The lesion is on the chest. A male subject aged 83 or over. The recorded lesion diameter is about 2.5 mm. This image is a 15 mm lesion crop taken from a total-body photograph. The tile uses white-light illumination.
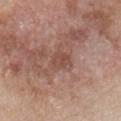Findings:
* biopsy diagnosis · a melanoma in situ — a malignant skin lesion